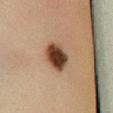<record>
  <biopsy_status>not biopsied; imaged during a skin examination</biopsy_status>
</record>